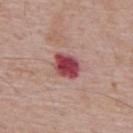follow-up: no biopsy performed (imaged during a skin exam) | subject: male, aged approximately 65 | acquisition: total-body-photography crop, ~15 mm field of view | diameter: ≈3.5 mm | lighting: white-light | anatomic site: the back.